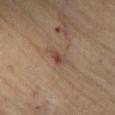Imaged during a routine full-body skin examination; the lesion was not biopsied and no histopathology is available.
Automated image analysis of the tile measured a lesion area of about 3 mm², an outline eccentricity of about 0.8 (0 = round, 1 = elongated), and two-axis asymmetry of about 0.35. The analysis additionally found a classifier nevus-likeness of about 0/100 and a detector confidence of about 100 out of 100 that the crop contains a lesion.
A male subject aged approximately 70.
A lesion tile, about 15 mm wide, cut from a 3D total-body photograph.
Imaged with cross-polarized lighting.
Longest diameter approximately 2.5 mm.
The lesion is on the front of the torso.